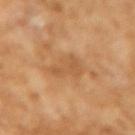Clinical impression:
No biopsy was performed on this lesion — it was imaged during a full skin examination and was not determined to be concerning.
Image and clinical context:
The lesion is located on the left forearm. A female patient, approximately 70 years of age. A close-up tile cropped from a whole-body skin photograph, about 15 mm across. This is a cross-polarized tile.